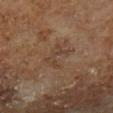Imaged during a routine full-body skin examination; the lesion was not biopsied and no histopathology is available.
A male subject aged 63–67.
The total-body-photography lesion software estimated an area of roughly 5.5 mm², an outline eccentricity of about 0.8 (0 = round, 1 = elongated), and two-axis asymmetry of about 0.5. And it measured an average lesion color of about L≈39 a*≈16 b*≈26 (CIELAB), roughly 5 lightness units darker than nearby skin, and a normalized lesion–skin contrast near 5. It also reported a classifier nevus-likeness of about 0/100 and a detector confidence of about 100 out of 100 that the crop contains a lesion.
The lesion's longest dimension is about 3.5 mm.
Imaged with cross-polarized lighting.
A lesion tile, about 15 mm wide, cut from a 3D total-body photograph.